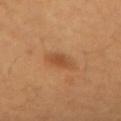The lesion was photographed on a routine skin check and not biopsied; there is no pathology result. A female subject, roughly 40 years of age. The lesion-visualizer software estimated a footprint of about 5 mm², an outline eccentricity of about 0.65 (0 = round, 1 = elongated), and a symmetry-axis asymmetry near 0.15. The software also gave a classifier nevus-likeness of about 65/100 and a detector confidence of about 100 out of 100 that the crop contains a lesion. Imaged with cross-polarized lighting. The lesion is located on the left forearm. A close-up tile cropped from a whole-body skin photograph, about 15 mm across.